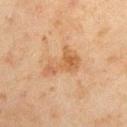Impression:
The lesion was tiled from a total-body skin photograph and was not biopsied.
Context:
On the right upper arm. A 15 mm close-up tile from a total-body photography series done for melanoma screening. A male patient aged approximately 45.